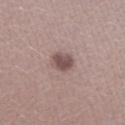Impression: The lesion was tiled from a total-body skin photograph and was not biopsied. Clinical summary: Automated image analysis of the tile measured a footprint of about 6 mm², an outline eccentricity of about 0.6 (0 = round, 1 = elongated), and two-axis asymmetry of about 0.15. The analysis additionally found a border-irregularity index near 1.5/10, a color-variation rating of about 2.5/10, and peripheral color asymmetry of about 1. The analysis additionally found an automated nevus-likeness rating near 60 out of 100. This image is a 15 mm lesion crop taken from a total-body photograph. On the right forearm. Captured under white-light illumination. A female patient, aged 43 to 47.